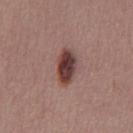Imaged during a routine full-body skin examination; the lesion was not biopsied and no histopathology is available.
A male patient aged 38–42.
Measured at roughly 4 mm in maximum diameter.
The tile uses white-light illumination.
A region of skin cropped from a whole-body photographic capture, roughly 15 mm wide.
The lesion is on the chest.